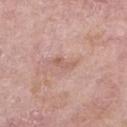Imaged during a routine full-body skin examination; the lesion was not biopsied and no histopathology is available.
A 15 mm crop from a total-body photograph taken for skin-cancer surveillance.
The lesion is on the arm.
About 3 mm across.
A female subject, aged approximately 70.
Automated image analysis of the tile measured an area of roughly 3 mm², an eccentricity of roughly 0.9, and a symmetry-axis asymmetry near 0.45. It also reported a classifier nevus-likeness of about 0/100 and a lesion-detection confidence of about 100/100.
This is a white-light tile.